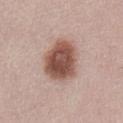Q: Was a biopsy performed?
A: total-body-photography surveillance lesion; no biopsy
Q: Lesion size?
A: about 5.5 mm
Q: What lighting was used for the tile?
A: white-light illumination
Q: What is the anatomic site?
A: the lower back
Q: Automated lesion metrics?
A: a lesion color around L≈52 a*≈20 b*≈25 in CIELAB, about 16 CIELAB-L* units darker than the surrounding skin, and a lesion-to-skin contrast of about 11 (normalized; higher = more distinct); a nevus-likeness score of about 100/100 and a detector confidence of about 100 out of 100 that the crop contains a lesion
Q: Who is the patient?
A: female, aged approximately 60
Q: How was this image acquired?
A: total-body-photography crop, ~15 mm field of view